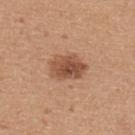biopsy status: no biopsy performed (imaged during a skin exam)
acquisition: ~15 mm crop, total-body skin-cancer survey
body site: the upper back
lighting: white-light illumination
subject: male, aged around 40
lesion size: ~4.5 mm (longest diameter)
image-analysis metrics: a lesion area of about 11 mm², an outline eccentricity of about 0.7 (0 = round, 1 = elongated), and a symmetry-axis asymmetry near 0.2; an average lesion color of about L≈51 a*≈22 b*≈32 (CIELAB), about 12 CIELAB-L* units darker than the surrounding skin, and a lesion-to-skin contrast of about 8.5 (normalized; higher = more distinct); a border-irregularity index near 2/10, internal color variation of about 5 on a 0–10 scale, and peripheral color asymmetry of about 1.5